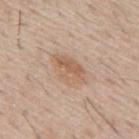<tbp_lesion>
<site>mid back</site>
<automated_metrics>
  <border_irregularity_0_10>2.5</border_irregularity_0_10>
  <color_variation_0_10>5.0</color_variation_0_10>
  <peripheral_color_asymmetry>1.5</peripheral_color_asymmetry>
  <nevus_likeness_0_100>40</nevus_likeness_0_100>
  <lesion_detection_confidence_0_100>100</lesion_detection_confidence_0_100>
</automated_metrics>
<patient>
  <sex>male</sex>
  <age_approx>55</age_approx>
</patient>
<image>
  <source>total-body photography crop</source>
  <field_of_view_mm>15</field_of_view_mm>
</image>
<lighting>white-light</lighting>
</tbp_lesion>